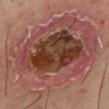This lesion was catalogued during total-body skin photography and was not selected for biopsy.
The lesion is on the mid back.
Automated image analysis of the tile measured a lesion area of about 100 mm². And it measured a mean CIELAB color near L≈42 a*≈23 b*≈24, roughly 11 lightness units darker than nearby skin, and a lesion-to-skin contrast of about 8.5 (normalized; higher = more distinct). The analysis additionally found border irregularity of about 3 on a 0–10 scale, internal color variation of about 9.5 on a 0–10 scale, and a peripheral color-asymmetry measure near 3. It also reported a nevus-likeness score of about 0/100 and a lesion-detection confidence of about 100/100.
The subject is a male about 40 years old.
Longest diameter approximately 12.5 mm.
A 15 mm crop from a total-body photograph taken for skin-cancer surveillance.
Captured under cross-polarized illumination.